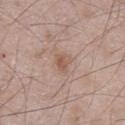Captured during whole-body skin photography for melanoma surveillance; the lesion was not biopsied. A male patient, aged 48 to 52. On the abdomen. A 15 mm close-up extracted from a 3D total-body photography capture.